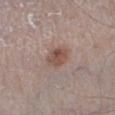Q: Was a biopsy performed?
A: catalogued during a skin exam; not biopsied
Q: What are the patient's age and sex?
A: male, aged 58 to 62
Q: What is the imaging modality?
A: ~15 mm tile from a whole-body skin photo
Q: Lesion location?
A: the left lower leg